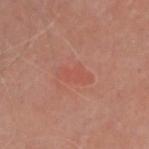follow-up: catalogued during a skin exam; not biopsied
patient: male, roughly 55 years of age
image: total-body-photography crop, ~15 mm field of view
automated metrics: a footprint of about 6 mm²; a lesion color around L≈53 a*≈28 b*≈30 in CIELAB and a lesion–skin lightness drop of about 5
anatomic site: the head or neck
illumination: cross-polarized illumination
size: about 4 mm